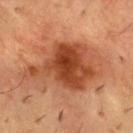follow-up: total-body-photography surveillance lesion; no biopsy
image source: 15 mm crop, total-body photography
lesion size: ≈9.5 mm
subject: male, about 55 years old
anatomic site: the front of the torso
illumination: cross-polarized illumination